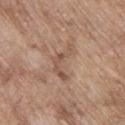<record>
<biopsy_status>not biopsied; imaged during a skin examination</biopsy_status>
<image>
  <source>total-body photography crop</source>
  <field_of_view_mm>15</field_of_view_mm>
</image>
<site>upper back</site>
<patient>
  <sex>male</sex>
  <age_approx>65</age_approx>
</patient>
<lesion_size>
  <long_diameter_mm_approx>5.0</long_diameter_mm_approx>
</lesion_size>
</record>